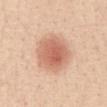notes = imaged on a skin check; not biopsied.